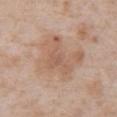{
  "image": {
    "source": "total-body photography crop",
    "field_of_view_mm": 15
  },
  "patient": {
    "sex": "male",
    "age_approx": 65
  },
  "site": "abdomen",
  "lesion_size": {
    "long_diameter_mm_approx": 5.5
  },
  "lighting": "white-light"
}